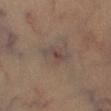Captured under cross-polarized illumination. The subject is a male in their mid- to late 40s. Automated tile analysis of the lesion measured an area of roughly 3.5 mm² and two-axis asymmetry of about 0.45. And it measured an average lesion color of about L≈40 a*≈13 b*≈19 (CIELAB), a lesion–skin lightness drop of about 6, and a normalized lesion–skin contrast near 6.5. It also reported an automated nevus-likeness rating near 0 out of 100. Located on the leg. A close-up tile cropped from a whole-body skin photograph, about 15 mm across. About 3.5 mm across.